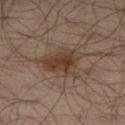Clinical impression: This lesion was catalogued during total-body skin photography and was not selected for biopsy. Clinical summary: A region of skin cropped from a whole-body photographic capture, roughly 15 mm wide. Located on the leg. A male patient approximately 65 years of age. This is a cross-polarized tile. An algorithmic analysis of the crop reported a footprint of about 12 mm², an outline eccentricity of about 0.7 (0 = round, 1 = elongated), and two-axis asymmetry of about 0.35. It also reported a border-irregularity rating of about 4/10, internal color variation of about 4.5 on a 0–10 scale, and a peripheral color-asymmetry measure near 1.5. It also reported a detector confidence of about 100 out of 100 that the crop contains a lesion.